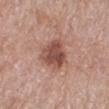The subject is a female roughly 55 years of age. A region of skin cropped from a whole-body photographic capture, roughly 15 mm wide. The lesion is located on the right lower leg. Approximately 4 mm at its widest. Imaged with white-light lighting.